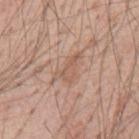Captured during whole-body skin photography for melanoma surveillance; the lesion was not biopsied. Located on the mid back. The patient is a male approximately 55 years of age. A roughly 15 mm field-of-view crop from a total-body skin photograph.